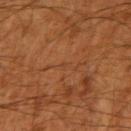Part of a total-body skin-imaging series; this lesion was reviewed on a skin check and was not flagged for biopsy. A region of skin cropped from a whole-body photographic capture, roughly 15 mm wide. Imaged with cross-polarized lighting. The lesion is on the right upper arm. A male patient, aged approximately 60. Longest diameter approximately 1 mm.